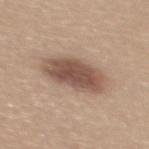– biopsy status · total-body-photography surveillance lesion; no biopsy
– patient · female, approximately 30 years of age
– imaging modality · ~15 mm crop, total-body skin-cancer survey
– lesion size · ≈6 mm
– lighting · white-light illumination
– location · the back